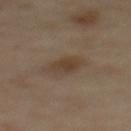A female patient approximately 60 years of age.
On the back.
This image is a 15 mm lesion crop taken from a total-body photograph.
Measured at roughly 3 mm in maximum diameter.
Automated image analysis of the tile measured an area of roughly 5 mm² and a symmetry-axis asymmetry near 0.15. And it measured a lesion–skin lightness drop of about 7. It also reported a border-irregularity index near 2/10, a within-lesion color-variation index near 3/10, and radial color variation of about 1.